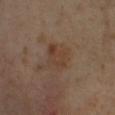Q: Was a biopsy performed?
A: catalogued during a skin exam; not biopsied
Q: How was this image acquired?
A: ~15 mm tile from a whole-body skin photo
Q: What is the anatomic site?
A: the leg
Q: What are the patient's age and sex?
A: female, roughly 55 years of age